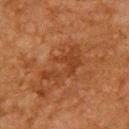{"biopsy_status": "not biopsied; imaged during a skin examination", "site": "back", "patient": {"sex": "male", "age_approx": 65}, "automated_metrics": {"area_mm2_approx": 13.0, "shape_asymmetry": 0.45, "lesion_detection_confidence_0_100": 100}, "image": {"source": "total-body photography crop", "field_of_view_mm": 15}, "lesion_size": {"long_diameter_mm_approx": 6.0}}